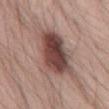This lesion was catalogued during total-body skin photography and was not selected for biopsy. This image is a 15 mm lesion crop taken from a total-body photograph. A male patient in their mid- to late 50s. Located on the abdomen. Measured at roughly 9 mm in maximum diameter. The tile uses white-light illumination.